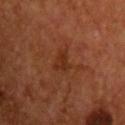Assessment:
Part of a total-body skin-imaging series; this lesion was reviewed on a skin check and was not flagged for biopsy.
Image and clinical context:
This is a cross-polarized tile. A close-up tile cropped from a whole-body skin photograph, about 15 mm across. A male subject aged 53–57. The lesion is on the chest.